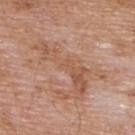biopsy_status: not biopsied; imaged during a skin examination
lesion_size:
  long_diameter_mm_approx: 8.0
site: upper back
automated_metrics:
  area_mm2_approx: 14.0
  eccentricity: 0.95
  cielab_L: 55
  cielab_a: 21
  cielab_b: 31
  vs_skin_darker_L: 7.0
  vs_skin_contrast_norm: 5.5
  border_irregularity_0_10: 10.0
  peripheral_color_asymmetry: 1.0
  nevus_likeness_0_100: 0
  lesion_detection_confidence_0_100: 100
patient:
  sex: male
  age_approx: 60
image:
  source: total-body photography crop
  field_of_view_mm: 15